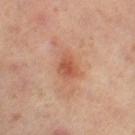{"patient": {"sex": "female", "age_approx": 40}, "automated_metrics": {"area_mm2_approx": 6.0, "eccentricity": 0.75, "shape_asymmetry": 0.3, "border_irregularity_0_10": 3.0, "color_variation_0_10": 3.5, "peripheral_color_asymmetry": 1.0, "nevus_likeness_0_100": 5}, "site": "right thigh", "lesion_size": {"long_diameter_mm_approx": 3.0}, "lighting": "cross-polarized", "image": {"source": "total-body photography crop", "field_of_view_mm": 15}}